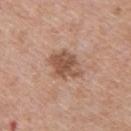biopsy_status: not biopsied; imaged during a skin examination
lesion_size:
  long_diameter_mm_approx: 4.0
image:
  source: total-body photography crop
  field_of_view_mm: 15
site: upper back
patient:
  sex: male
  age_approx: 60
automated_metrics:
  area_mm2_approx: 9.5
  eccentricity: 0.65
  border_irregularity_0_10: 3.5
  color_variation_0_10: 3.0
  peripheral_color_asymmetry: 1.0
  nevus_likeness_0_100: 0
  lesion_detection_confidence_0_100: 100